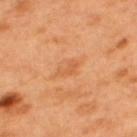workup: catalogued during a skin exam; not biopsied
TBP lesion metrics: a lesion color around L≈59 a*≈27 b*≈42 in CIELAB and a lesion–skin lightness drop of about 7
image source: 15 mm crop, total-body photography
lesion size: ~3 mm (longest diameter)
illumination: cross-polarized illumination
body site: the upper back
patient: male, aged around 50